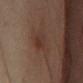<lesion>
<biopsy_status>not biopsied; imaged during a skin examination</biopsy_status>
<lighting>cross-polarized</lighting>
<patient>
  <sex>female</sex>
  <age_approx>55</age_approx>
</patient>
<lesion_size>
  <long_diameter_mm_approx>6.0</long_diameter_mm_approx>
</lesion_size>
<automated_metrics>
  <area_mm2_approx>11.0</area_mm2_approx>
  <eccentricity>0.9</eccentricity>
  <shape_asymmetry>0.3</shape_asymmetry>
  <cielab_L>28</cielab_L>
  <cielab_a>14</cielab_a>
  <cielab_b>20</cielab_b>
  <vs_skin_darker_L>4.0</vs_skin_darker_L>
  <border_irregularity_0_10>4.0</border_irregularity_0_10>
  <color_variation_0_10>3.0</color_variation_0_10>
  <nevus_likeness_0_100>5</nevus_likeness_0_100>
  <lesion_detection_confidence_0_100>100</lesion_detection_confidence_0_100>
</automated_metrics>
<site>abdomen</site>
<image>
  <source>total-body photography crop</source>
  <field_of_view_mm>15</field_of_view_mm>
</image>
</lesion>